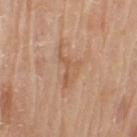Impression: The lesion was photographed on a routine skin check and not biopsied; there is no pathology result. Clinical summary: A male patient, aged around 80. A 15 mm crop from a total-body photograph taken for skin-cancer surveillance. The lesion is located on the right upper arm. This is a white-light tile.